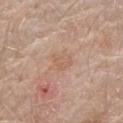Clinical impression: Recorded during total-body skin imaging; not selected for excision or biopsy. Clinical summary: Automated tile analysis of the lesion measured an average lesion color of about L≈60 a*≈19 b*≈31 (CIELAB), about 5 CIELAB-L* units darker than the surrounding skin, and a normalized border contrast of about 4.5. The analysis additionally found a border-irregularity index near 2.5/10 and peripheral color asymmetry of about 1. And it measured a nevus-likeness score of about 0/100 and a lesion-detection confidence of about 100/100. A male patient in their 70s. A 15 mm close-up extracted from a 3D total-body photography capture. Located on the right forearm. Longest diameter approximately 3 mm.